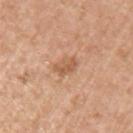biopsy_status: not biopsied; imaged during a skin examination
patient:
  sex: male
  age_approx: 70
site: left upper arm
image:
  source: total-body photography crop
  field_of_view_mm: 15